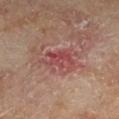Case summary:
* notes — imaged on a skin check; not biopsied
* anatomic site — the leg
* tile lighting — cross-polarized illumination
* image source — ~15 mm crop, total-body skin-cancer survey
* lesion diameter — about 4 mm
* automated metrics — a shape eccentricity near 0.75 and a symmetry-axis asymmetry near 0.4; an automated nevus-likeness rating near 0 out of 100 and a lesion-detection confidence of about 100/100
* patient — male, in their mid- to late 60s